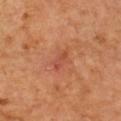No biopsy was performed on this lesion — it was imaged during a full skin examination and was not determined to be concerning. Located on the arm. A 15 mm crop from a total-body photograph taken for skin-cancer surveillance. Measured at roughly 2.5 mm in maximum diameter. The subject is a male approximately 60 years of age. The tile uses cross-polarized illumination.